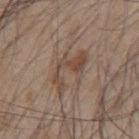Assessment: Captured during whole-body skin photography for melanoma surveillance; the lesion was not biopsied. Background: Cropped from a whole-body photographic skin survey; the tile spans about 15 mm. On the mid back. A male subject, aged around 45. The total-body-photography lesion software estimated a footprint of about 9.5 mm² and a shape eccentricity near 0.85. The software also gave a nevus-likeness score of about 5/100. About 6 mm across. Captured under white-light illumination.